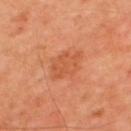Findings:
- biopsy status: catalogued during a skin exam; not biopsied
- site: the upper back
- size: about 4 mm
- image: total-body-photography crop, ~15 mm field of view
- subject: male, aged 48–52
- tile lighting: cross-polarized illumination